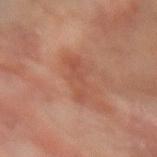Recorded during total-body skin imaging; not selected for excision or biopsy.
The patient is a female aged around 80.
Measured at roughly 4 mm in maximum diameter.
From the left forearm.
A lesion tile, about 15 mm wide, cut from a 3D total-body photograph.
The tile uses cross-polarized illumination.
Automated tile analysis of the lesion measured an outline eccentricity of about 0.85 (0 = round, 1 = elongated) and a symmetry-axis asymmetry near 0.35. And it measured a lesion color around L≈43 a*≈22 b*≈27 in CIELAB, a lesion–skin lightness drop of about 6, and a normalized border contrast of about 4.5. It also reported a border-irregularity index near 4.5/10, a color-variation rating of about 2.5/10, and peripheral color asymmetry of about 1. The analysis additionally found a classifier nevus-likeness of about 0/100.